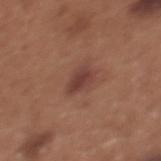Assessment: The lesion was tiled from a total-body skin photograph and was not biopsied. Acquisition and patient details: Imaged with white-light lighting. The lesion's longest dimension is about 3 mm. The total-body-photography lesion software estimated a lesion area of about 5.5 mm², an eccentricity of roughly 0.75, and two-axis asymmetry of about 0.25. The analysis additionally found a lesion color around L≈40 a*≈22 b*≈24 in CIELAB, about 9 CIELAB-L* units darker than the surrounding skin, and a lesion-to-skin contrast of about 8 (normalized; higher = more distinct). A 15 mm close-up extracted from a 3D total-body photography capture. The lesion is on the chest. The patient is a female aged 33 to 37.